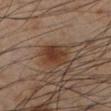<record>
<biopsy_status>not biopsied; imaged during a skin examination</biopsy_status>
<site>left lower leg</site>
<automated_metrics>
  <area_mm2_approx>9.5</area_mm2_approx>
  <eccentricity>0.6</eccentricity>
  <shape_asymmetry>0.2</shape_asymmetry>
  <cielab_L>38</cielab_L>
  <cielab_a>18</cielab_a>
  <cielab_b>28</cielab_b>
  <vs_skin_darker_L>9.0</vs_skin_darker_L>
  <vs_skin_contrast_norm>8.5</vs_skin_contrast_norm>
  <border_irregularity_0_10>2.0</border_irregularity_0_10>
  <peripheral_color_asymmetry>1.5</peripheral_color_asymmetry>
</automated_metrics>
<lesion_size>
  <long_diameter_mm_approx>4.0</long_diameter_mm_approx>
</lesion_size>
<patient>
  <sex>male</sex>
  <age_approx>55</age_approx>
</patient>
<image>
  <source>total-body photography crop</source>
  <field_of_view_mm>15</field_of_view_mm>
</image>
<lighting>cross-polarized</lighting>
</record>